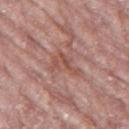Assessment: Imaged during a routine full-body skin examination; the lesion was not biopsied and no histopathology is available. Image and clinical context: The lesion is located on the left thigh. The tile uses white-light illumination. This image is a 15 mm lesion crop taken from a total-body photograph. The patient is a female aged 73 to 77. Longest diameter approximately 4 mm. Automated image analysis of the tile measured a lesion color around L≈52 a*≈23 b*≈26 in CIELAB, a lesion–skin lightness drop of about 7, and a lesion-to-skin contrast of about 6 (normalized; higher = more distinct). The software also gave a border-irregularity index near 6.5/10, a color-variation rating of about 5/10, and radial color variation of about 2.